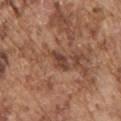• biopsy status · no biopsy performed (imaged during a skin exam)
• image · ~15 mm tile from a whole-body skin photo
• site · the chest
• patient · male, approximately 75 years of age